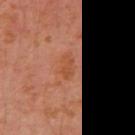This lesion was catalogued during total-body skin photography and was not selected for biopsy. A 15 mm close-up extracted from a 3D total-body photography capture. On the left arm. The tile uses cross-polarized illumination. A male patient, aged 28 to 32.